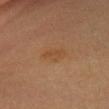Clinical impression: Recorded during total-body skin imaging; not selected for excision or biopsy. Background: The lesion's longest dimension is about 3 mm. On the head or neck. A close-up tile cropped from a whole-body skin photograph, about 15 mm across. Automated image analysis of the tile measured a footprint of about 4.5 mm², an eccentricity of roughly 0.85, and a symmetry-axis asymmetry near 0.2. The analysis additionally found border irregularity of about 2.5 on a 0–10 scale, internal color variation of about 1.5 on a 0–10 scale, and radial color variation of about 0.5. The software also gave an automated nevus-likeness rating near 10 out of 100 and a detector confidence of about 100 out of 100 that the crop contains a lesion. The tile uses cross-polarized illumination. A female patient in their mid- to late 40s.